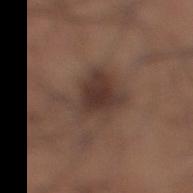No biopsy was performed on this lesion — it was imaged during a full skin examination and was not determined to be concerning.
Captured under white-light illumination.
A male patient, approximately 35 years of age.
A 15 mm close-up tile from a total-body photography series done for melanoma screening.
The lesion is on the right lower leg.
The lesion's longest dimension is about 7 mm.